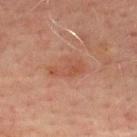notes=imaged on a skin check; not biopsied
lesion size=about 4 mm
site=the chest
imaging modality=15 mm crop, total-body photography
tile lighting=cross-polarized
subject=male, in their 70s